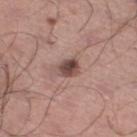  lesion_size:
    long_diameter_mm_approx: 3.0
  site: leg
  automated_metrics:
    area_mm2_approx: 5.0
    eccentricity: 0.55
    cielab_L: 47
    cielab_a: 19
    cielab_b: 22
    vs_skin_darker_L: 14.0
    vs_skin_contrast_norm: 10.0
    border_irregularity_0_10: 2.5
    color_variation_0_10: 5.5
  image:
    source: total-body photography crop
    field_of_view_mm: 15
  patient:
    sex: male
    age_approx: 40
  lighting: white-light